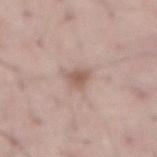Q: Was this lesion biopsied?
A: no biopsy performed (imaged during a skin exam)
Q: What kind of image is this?
A: ~15 mm tile from a whole-body skin photo
Q: How large is the lesion?
A: ~2.5 mm (longest diameter)
Q: What is the anatomic site?
A: the front of the torso
Q: How was the tile lit?
A: white-light
Q: Who is the patient?
A: male, roughly 65 years of age